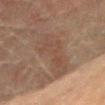Assessment:
No biopsy was performed on this lesion — it was imaged during a full skin examination and was not determined to be concerning.
Acquisition and patient details:
A male subject roughly 75 years of age. Imaged with cross-polarized lighting. The lesion is on the abdomen. A lesion tile, about 15 mm wide, cut from a 3D total-body photograph. About 7 mm across.